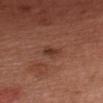A female patient, aged approximately 60.
Imaged with white-light lighting.
On the chest.
A region of skin cropped from a whole-body photographic capture, roughly 15 mm wide.